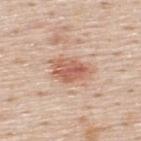Case summary:
• image source · ~15 mm crop, total-body skin-cancer survey
• anatomic site · the back
• automated metrics · an outline eccentricity of about 0.8 (0 = round, 1 = elongated) and two-axis asymmetry of about 0.25; a mean CIELAB color near L≈61 a*≈23 b*≈30; internal color variation of about 5 on a 0–10 scale and radial color variation of about 1.5
• subject · male, aged approximately 45
• lesion diameter · ≈5 mm